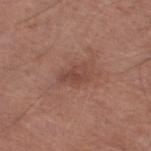subject — male, aged around 70
diameter — ≈3 mm
automated metrics — a classifier nevus-likeness of about 0/100 and a lesion-detection confidence of about 100/100
acquisition — 15 mm crop, total-body photography
illumination — white-light illumination
site — the left lower leg